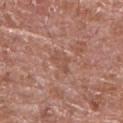<tbp_lesion>
<biopsy_status>not biopsied; imaged during a skin examination</biopsy_status>
<lesion_size>
  <long_diameter_mm_approx>3.0</long_diameter_mm_approx>
</lesion_size>
<site>right upper arm</site>
<patient>
  <sex>male</sex>
  <age_approx>80</age_approx>
</patient>
<lighting>white-light</lighting>
<image>
  <source>total-body photography crop</source>
  <field_of_view_mm>15</field_of_view_mm>
</image>
</tbp_lesion>